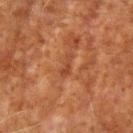On the right upper arm. A male patient, aged around 70. A 15 mm crop from a total-body photograph taken for skin-cancer surveillance. An algorithmic analysis of the crop reported a lesion area of about 2.5 mm², a shape eccentricity near 0.95, and two-axis asymmetry of about 0.35. And it measured an average lesion color of about L≈37 a*≈24 b*≈31 (CIELAB) and a lesion-to-skin contrast of about 6 (normalized; higher = more distinct).